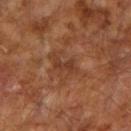{"biopsy_status": "not biopsied; imaged during a skin examination", "site": "right upper arm", "image": {"source": "total-body photography crop", "field_of_view_mm": 15}, "automated_metrics": {"cielab_L": 36, "cielab_a": 22, "cielab_b": 30, "vs_skin_darker_L": 7.0, "vs_skin_contrast_norm": 6.0, "border_irregularity_0_10": 5.5, "color_variation_0_10": 0.0, "lesion_detection_confidence_0_100": 95}, "lighting": "cross-polarized", "patient": {"sex": "male", "age_approx": 65}, "lesion_size": {"long_diameter_mm_approx": 2.5}}